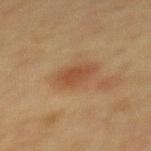Clinical impression:
The lesion was tiled from a total-body skin photograph and was not biopsied.
Background:
The lesion is located on the back. Captured under cross-polarized illumination. Cropped from a whole-body photographic skin survey; the tile spans about 15 mm. A female patient, aged 48 to 52.